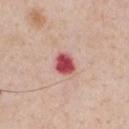Background:
A male patient, about 60 years old. The lesion is on the chest. The tile uses white-light illumination. Approximately 2.5 mm at its widest. The total-body-photography lesion software estimated a lesion color around L≈50 a*≈38 b*≈23 in CIELAB, about 20 CIELAB-L* units darker than the surrounding skin, and a normalized lesion–skin contrast near 13. It also reported peripheral color asymmetry of about 1. A region of skin cropped from a whole-body photographic capture, roughly 15 mm wide.